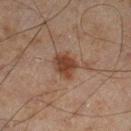Assessment:
This lesion was catalogued during total-body skin photography and was not selected for biopsy.
Image and clinical context:
Located on the right lower leg. This image is a 15 mm lesion crop taken from a total-body photograph. Imaged with cross-polarized lighting. The recorded lesion diameter is about 3 mm. An algorithmic analysis of the crop reported an average lesion color of about L≈33 a*≈17 b*≈24 (CIELAB) and a normalized lesion–skin contrast near 9. The software also gave a classifier nevus-likeness of about 85/100 and a detector confidence of about 100 out of 100 that the crop contains a lesion. A male subject roughly 45 years of age.